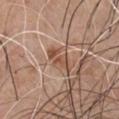Clinical impression:
This lesion was catalogued during total-body skin photography and was not selected for biopsy.
Acquisition and patient details:
A male subject aged around 65. About 4 mm across. A close-up tile cropped from a whole-body skin photograph, about 15 mm across. On the front of the torso.